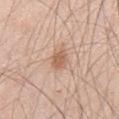| field | value |
|---|---|
| workup | catalogued during a skin exam; not biopsied |
| subject | male, in their mid-40s |
| location | the chest |
| image source | 15 mm crop, total-body photography |
| illumination | white-light illumination |
| diameter | about 2.5 mm |